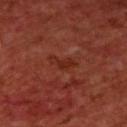location — the upper back
diameter — ~3 mm (longest diameter)
illumination — cross-polarized illumination
image — ~15 mm crop, total-body skin-cancer survey
subject — male, roughly 60 years of age